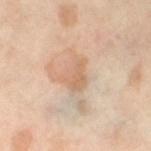biopsy status = no biopsy performed (imaged during a skin exam)
lesion size = ~3.5 mm (longest diameter)
image-analysis metrics = a border-irregularity index near 4.5/10, a color-variation rating of about 2.5/10, and radial color variation of about 1; a classifier nevus-likeness of about 15/100 and a lesion-detection confidence of about 95/100
patient = female, aged 48 to 52
image source = ~15 mm tile from a whole-body skin photo
body site = the left thigh